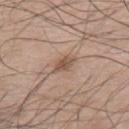No biopsy was performed on this lesion — it was imaged during a full skin examination and was not determined to be concerning.
The tile uses white-light illumination.
A male subject, aged around 75.
Automated image analysis of the tile measured an outline eccentricity of about 0.85 (0 = round, 1 = elongated) and a symmetry-axis asymmetry near 0.35.
The lesion is on the mid back.
A lesion tile, about 15 mm wide, cut from a 3D total-body photograph.